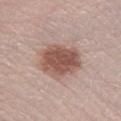The lesion was tiled from a total-body skin photograph and was not biopsied.
This is a white-light tile.
Longest diameter approximately 5.5 mm.
A close-up tile cropped from a whole-body skin photograph, about 15 mm across.
A female patient, aged approximately 40.
The lesion is located on the right lower leg.